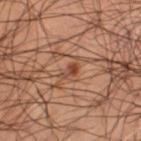- illumination — cross-polarized illumination
- body site — the left thigh
- image source — 15 mm crop, total-body photography
- size — about 2.5 mm
- patient — male, approximately 65 years of age
- automated lesion analysis — internal color variation of about 1.5 on a 0–10 scale; an automated nevus-likeness rating near 95 out of 100 and a lesion-detection confidence of about 100/100